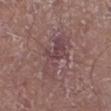| field | value |
|---|---|
| follow-up | catalogued during a skin exam; not biopsied |
| image | ~15 mm crop, total-body skin-cancer survey |
| patient | male, aged 68–72 |
| illumination | white-light |
| anatomic site | the leg |
| lesion size | about 5.5 mm |
| automated metrics | a lesion color around L≈44 a*≈20 b*≈16 in CIELAB, roughly 7 lightness units darker than nearby skin, and a normalized lesion–skin contrast near 6 |